A lesion tile, about 15 mm wide, cut from a 3D total-body photograph · the tile uses white-light illumination · a female patient, aged approximately 70 · on the leg · about 8.5 mm across · The total-body-photography lesion software estimated an area of roughly 36 mm², a shape eccentricity near 0.65, and two-axis asymmetry of about 0.45. The software also gave a border-irregularity rating of about 5/10 and a peripheral color-asymmetry measure near 2.
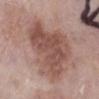On biopsy, histopathology showed a malignant skin lesion: melanoma in situ.